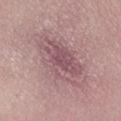  biopsy_status: not biopsied; imaged during a skin examination
  automated_metrics:
    vs_skin_darker_L: 9.0
    vs_skin_contrast_norm: 6.5
    nevus_likeness_0_100: 0
    lesion_detection_confidence_0_100: 95
  patient:
    sex: female
    age_approx: 20
  lighting: white-light
  lesion_size:
    long_diameter_mm_approx: 8.0
  site: right lower leg
  image:
    source: total-body photography crop
    field_of_view_mm: 15